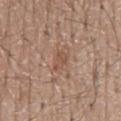{"biopsy_status": "not biopsied; imaged during a skin examination", "image": {"source": "total-body photography crop", "field_of_view_mm": 15}, "lesion_size": {"long_diameter_mm_approx": 2.5}, "patient": {"sex": "male", "age_approx": 65}, "site": "mid back", "lighting": "white-light", "automated_metrics": {"cielab_L": 51, "cielab_a": 19, "cielab_b": 28, "vs_skin_contrast_norm": 5.5}}